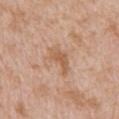{
  "biopsy_status": "not biopsied; imaged during a skin examination",
  "patient": {
    "sex": "male",
    "age_approx": 60
  },
  "image": {
    "source": "total-body photography crop",
    "field_of_view_mm": 15
  },
  "lesion_size": {
    "long_diameter_mm_approx": 4.0
  },
  "lighting": "white-light",
  "site": "mid back",
  "automated_metrics": {
    "area_mm2_approx": 6.0,
    "shape_asymmetry": 0.45,
    "cielab_L": 59,
    "cielab_a": 20,
    "cielab_b": 33,
    "vs_skin_darker_L": 8.0,
    "vs_skin_contrast_norm": 6.5,
    "nevus_likeness_0_100": 0,
    "lesion_detection_confidence_0_100": 100
  }
}